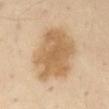This lesion was catalogued during total-body skin photography and was not selected for biopsy.
The patient is a male aged approximately 55.
The lesion's longest dimension is about 8 mm.
The lesion is located on the abdomen.
A roughly 15 mm field-of-view crop from a total-body skin photograph.
Automated tile analysis of the lesion measured an area of roughly 33 mm², an eccentricity of roughly 0.65, and a shape-asymmetry score of about 0.2 (0 = symmetric). The software also gave a lesion–skin lightness drop of about 11 and a lesion-to-skin contrast of about 8 (normalized; higher = more distinct). The analysis additionally found an automated nevus-likeness rating near 15 out of 100.